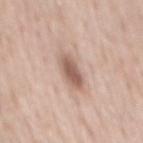Assessment: Part of a total-body skin-imaging series; this lesion was reviewed on a skin check and was not flagged for biopsy. Acquisition and patient details: The total-body-photography lesion software estimated border irregularity of about 2 on a 0–10 scale. A male patient, approximately 50 years of age. Longest diameter approximately 4 mm. A roughly 15 mm field-of-view crop from a total-body skin photograph. On the mid back. Captured under white-light illumination.